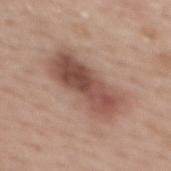biopsy status: no biopsy performed (imaged during a skin exam); image source: ~15 mm tile from a whole-body skin photo; site: the upper back; patient: female, aged 58–62.